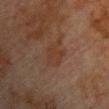notes: total-body-photography surveillance lesion; no biopsy | anatomic site: the chest | subject: male, aged around 75 | imaging modality: 15 mm crop, total-body photography | tile lighting: cross-polarized | diameter: ~4 mm (longest diameter).